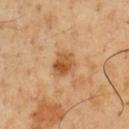Captured under cross-polarized illumination. Longest diameter approximately 3.5 mm. The lesion is located on the front of the torso. A male patient, in their 50s. Cropped from a total-body skin-imaging series; the visible field is about 15 mm.